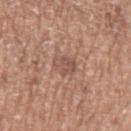biopsy_status: not biopsied; imaged during a skin examination
image:
  source: total-body photography crop
  field_of_view_mm: 15
lighting: white-light
site: left upper arm
patient:
  sex: male
  age_approx: 75
lesion_size:
  long_diameter_mm_approx: 2.5
automated_metrics:
  area_mm2_approx: 4.5
  cielab_L: 52
  cielab_a: 20
  cielab_b: 25
  vs_skin_contrast_norm: 6.0
  nevus_likeness_0_100: 0
  lesion_detection_confidence_0_100: 85